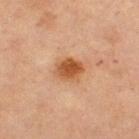Imaged during a routine full-body skin examination; the lesion was not biopsied and no histopathology is available.
Located on the left thigh.
The total-body-photography lesion software estimated a shape eccentricity near 0.55 and a shape-asymmetry score of about 0.15 (0 = symmetric). The analysis additionally found a nevus-likeness score of about 95/100 and a detector confidence of about 100 out of 100 that the crop contains a lesion.
A female patient approximately 70 years of age.
Captured under cross-polarized illumination.
Cropped from a whole-body photographic skin survey; the tile spans about 15 mm.
Measured at roughly 3.5 mm in maximum diameter.